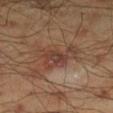Image and clinical context:
Imaged with cross-polarized lighting. A male patient, in their mid-40s. Cropped from a total-body skin-imaging series; the visible field is about 15 mm. Approximately 5.5 mm at its widest. Automated image analysis of the tile measured an area of roughly 12 mm², an outline eccentricity of about 0.8 (0 = round, 1 = elongated), and a shape-asymmetry score of about 0.45 (0 = symmetric). The software also gave a mean CIELAB color near L≈40 a*≈19 b*≈26 and a normalized border contrast of about 7. The analysis additionally found a lesion-detection confidence of about 100/100. From the right lower leg.